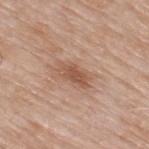Imaged during a routine full-body skin examination; the lesion was not biopsied and no histopathology is available. The lesion is located on the upper back. A male subject, aged 63 to 67. A 15 mm crop from a total-body photograph taken for skin-cancer surveillance. Longest diameter approximately 3.5 mm. The lesion-visualizer software estimated an average lesion color of about L≈53 a*≈21 b*≈30 (CIELAB). And it measured a classifier nevus-likeness of about 20/100. The tile uses white-light illumination.